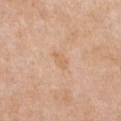notes=total-body-photography surveillance lesion; no biopsy | diameter=≈2.5 mm | image source=total-body-photography crop, ~15 mm field of view | tile lighting=white-light | site=the chest | patient=female, aged 63–67.